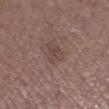workup: catalogued during a skin exam; not biopsied
patient: male, about 75 years old
anatomic site: the left lower leg
automated lesion analysis: a lesion area of about 3.5 mm² and a symmetry-axis asymmetry near 0.3; about 6 CIELAB-L* units darker than the surrounding skin; internal color variation of about 1.5 on a 0–10 scale
image: total-body-photography crop, ~15 mm field of view
lesion size: ≈2.5 mm